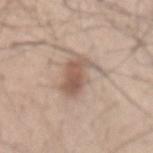{
  "biopsy_status": "not biopsied; imaged during a skin examination",
  "image": {
    "source": "total-body photography crop",
    "field_of_view_mm": 15
  },
  "site": "lower back",
  "patient": {
    "sex": "male",
    "age_approx": 45
  }
}